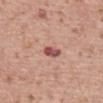| field | value |
|---|---|
| notes | imaged on a skin check; not biopsied |
| tile lighting | white-light |
| image source | ~15 mm crop, total-body skin-cancer survey |
| diameter | about 2.5 mm |
| subject | male, aged around 70 |
| site | the mid back |